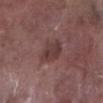biopsy status: catalogued during a skin exam; not biopsied
subject: male, in their mid-70s
illumination: white-light illumination
image source: 15 mm crop, total-body photography
diameter: ≈3 mm
anatomic site: the right lower leg